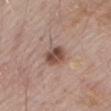workup = imaged on a skin check; not biopsied | patient = male, about 70 years old | automated metrics = border irregularity of about 1.5 on a 0–10 scale, internal color variation of about 5.5 on a 0–10 scale, and peripheral color asymmetry of about 2; an automated nevus-likeness rating near 80 out of 100 and a lesion-detection confidence of about 100/100 | anatomic site = the mid back | image source = ~15 mm crop, total-body skin-cancer survey | illumination = white-light.